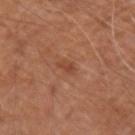workup — no biopsy performed (imaged during a skin exam)
TBP lesion metrics — a lesion color around L≈45 a*≈25 b*≈33 in CIELAB, about 8 CIELAB-L* units darker than the surrounding skin, and a normalized border contrast of about 6; a nevus-likeness score of about 0/100 and a lesion-detection confidence of about 100/100
tile lighting — white-light
subject — male, aged 68–72
diameter — ≈2.5 mm
image — 15 mm crop, total-body photography
site — the right upper arm